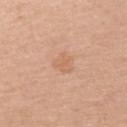The lesion was tiled from a total-body skin photograph and was not biopsied. Imaged with white-light lighting. The lesion is on the left upper arm. A lesion tile, about 15 mm wide, cut from a 3D total-body photograph. Automated image analysis of the tile measured a shape eccentricity near 0.35 and two-axis asymmetry of about 0.25. It also reported an average lesion color of about L≈64 a*≈21 b*≈34 (CIELAB), about 6 CIELAB-L* units darker than the surrounding skin, and a normalized lesion–skin contrast near 4.5. The analysis additionally found a classifier nevus-likeness of about 0/100 and a lesion-detection confidence of about 100/100. The patient is a female approximately 50 years of age.